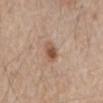The lesion was photographed on a routine skin check and not biopsied; there is no pathology result. Approximately 3 mm at its widest. The patient is a male aged around 80. Imaged with white-light lighting. The lesion is located on the front of the torso. A 15 mm crop from a total-body photograph taken for skin-cancer surveillance.